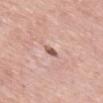Context:
A male subject aged approximately 55. Located on the mid back. A roughly 15 mm field-of-view crop from a total-body skin photograph.